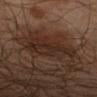Imaged during a routine full-body skin examination; the lesion was not biopsied and no histopathology is available.
A roughly 15 mm field-of-view crop from a total-body skin photograph.
About 5.5 mm across.
Automated tile analysis of the lesion measured a shape-asymmetry score of about 0.3 (0 = symmetric). The analysis additionally found an average lesion color of about L≈24 a*≈15 b*≈21 (CIELAB), roughly 6 lightness units darker than nearby skin, and a lesion-to-skin contrast of about 7.5 (normalized; higher = more distinct).
On the right upper arm.
The tile uses cross-polarized illumination.
The subject is a male aged approximately 65.